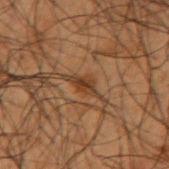workup — total-body-photography surveillance lesion; no biopsy
body site — the arm
diameter — ~3 mm (longest diameter)
image-analysis metrics — a footprint of about 3.5 mm², an eccentricity of roughly 0.85, and two-axis asymmetry of about 0.25; roughly 7 lightness units darker than nearby skin; a border-irregularity rating of about 3.5/10 and a peripheral color-asymmetry measure near 1; a detector confidence of about 95 out of 100 that the crop contains a lesion
subject — male, roughly 50 years of age
lighting — cross-polarized
acquisition — 15 mm crop, total-body photography